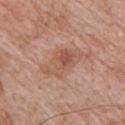{
  "biopsy_status": "not biopsied; imaged during a skin examination",
  "automated_metrics": {
    "cielab_L": 54,
    "cielab_a": 22,
    "cielab_b": 29,
    "vs_skin_darker_L": 8.0,
    "vs_skin_contrast_norm": 6.0,
    "border_irregularity_0_10": 5.5,
    "color_variation_0_10": 5.0,
    "peripheral_color_asymmetry": 1.5
  },
  "lesion_size": {
    "long_diameter_mm_approx": 5.0
  },
  "lighting": "white-light",
  "site": "chest",
  "patient": {
    "sex": "male",
    "age_approx": 70
  },
  "image": {
    "source": "total-body photography crop",
    "field_of_view_mm": 15
  }
}